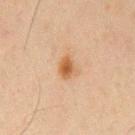The lesion was photographed on a routine skin check and not biopsied; there is no pathology result. A male subject aged 63 to 67. The lesion is located on the chest. A lesion tile, about 15 mm wide, cut from a 3D total-body photograph.